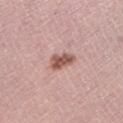Imaged during a routine full-body skin examination; the lesion was not biopsied and no histopathology is available. The lesion is on the left lower leg. A female patient in their mid-60s. Approximately 3 mm at its widest. A lesion tile, about 15 mm wide, cut from a 3D total-body photograph. Imaged with white-light lighting.